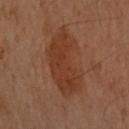Q: Was this lesion biopsied?
A: no biopsy performed (imaged during a skin exam)
Q: Lesion location?
A: the front of the torso
Q: What is the imaging modality?
A: ~15 mm crop, total-body skin-cancer survey
Q: Patient demographics?
A: female, aged approximately 60